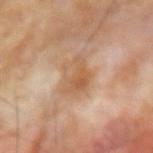No biopsy was performed on this lesion — it was imaged during a full skin examination and was not determined to be concerning. A male patient about 70 years old. An algorithmic analysis of the crop reported a border-irregularity index near 3/10 and a within-lesion color-variation index near 5.5/10. The lesion's longest dimension is about 4 mm. A region of skin cropped from a whole-body photographic capture, roughly 15 mm wide. The lesion is on the arm.